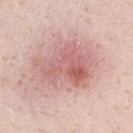Captured during whole-body skin photography for melanoma surveillance; the lesion was not biopsied. A male subject, aged approximately 35. Cropped from a total-body skin-imaging series; the visible field is about 15 mm. Located on the left upper arm.